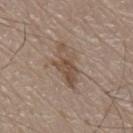No biopsy was performed on this lesion — it was imaged during a full skin examination and was not determined to be concerning. This is a white-light tile. Automated image analysis of the tile measured an average lesion color of about L≈49 a*≈15 b*≈26 (CIELAB), roughly 9 lightness units darker than nearby skin, and a normalized border contrast of about 7.5. The software also gave border irregularity of about 5.5 on a 0–10 scale, a within-lesion color-variation index near 2/10, and radial color variation of about 0.5. A male patient, aged 78 to 82. Located on the chest. Cropped from a total-body skin-imaging series; the visible field is about 15 mm.